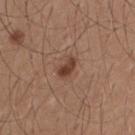Acquisition and patient details:
The tile uses white-light illumination. An algorithmic analysis of the crop reported an eccentricity of roughly 0.8 and a shape-asymmetry score of about 0.25 (0 = symmetric). It also reported a mean CIELAB color near L≈41 a*≈20 b*≈27, about 11 CIELAB-L* units darker than the surrounding skin, and a normalized lesion–skin contrast near 9. The analysis additionally found an automated nevus-likeness rating near 90 out of 100 and a lesion-detection confidence of about 100/100. The lesion is located on the upper back. Cropped from a whole-body photographic skin survey; the tile spans about 15 mm. A male subject, aged around 25. About 2.5 mm across.